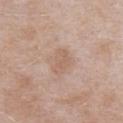  biopsy_status: not biopsied; imaged during a skin examination